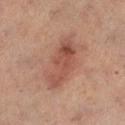Q: Automated lesion metrics?
A: roughly 9 lightness units darker than nearby skin and a normalized lesion–skin contrast near 7; border irregularity of about 4 on a 0–10 scale, a within-lesion color-variation index near 4.5/10, and radial color variation of about 1.5; an automated nevus-likeness rating near 85 out of 100 and a detector confidence of about 100 out of 100 that the crop contains a lesion
Q: Who is the patient?
A: female, aged around 55
Q: Lesion size?
A: about 6.5 mm
Q: What kind of image is this?
A: ~15 mm crop, total-body skin-cancer survey
Q: Where on the body is the lesion?
A: the left lower leg